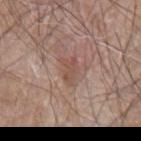<case>
<biopsy_status>not biopsied; imaged during a skin examination</biopsy_status>
<patient>
  <sex>male</sex>
  <age_approx>80</age_approx>
</patient>
<automated_metrics>
  <area_mm2_approx>5.0</area_mm2_approx>
  <eccentricity>0.8</eccentricity>
  <shape_asymmetry>0.35</shape_asymmetry>
  <vs_skin_contrast_norm>5.5</vs_skin_contrast_norm>
</automated_metrics>
<lesion_size>
  <long_diameter_mm_approx>3.5</long_diameter_mm_approx>
</lesion_size>
<site>left arm</site>
<lighting>white-light</lighting>
<image>
  <source>total-body photography crop</source>
  <field_of_view_mm>15</field_of_view_mm>
</image>
</case>